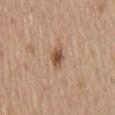Findings:
- notes · no biopsy performed (imaged during a skin exam)
- illumination · white-light
- imaging modality · 15 mm crop, total-body photography
- subject · male, aged 68 to 72
- location · the mid back
- lesion diameter · about 3 mm